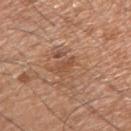The lesion was tiled from a total-body skin photograph and was not biopsied. A male patient, approximately 65 years of age. This is a white-light tile. On the right upper arm. A roughly 15 mm field-of-view crop from a total-body skin photograph. The recorded lesion diameter is about 2.5 mm. Automated tile analysis of the lesion measured a lesion color around L≈50 a*≈22 b*≈33 in CIELAB and about 7 CIELAB-L* units darker than the surrounding skin. It also reported a border-irregularity index near 3.5/10, internal color variation of about 0 on a 0–10 scale, and peripheral color asymmetry of about 0.